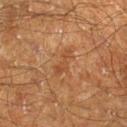This lesion was catalogued during total-body skin photography and was not selected for biopsy. The lesion is on the right lower leg. Automated image analysis of the tile measured a border-irregularity index near 6/10, internal color variation of about 1 on a 0–10 scale, and a peripheral color-asymmetry measure near 0. The software also gave lesion-presence confidence of about 100/100. A roughly 15 mm field-of-view crop from a total-body skin photograph. Measured at roughly 3.5 mm in maximum diameter. The patient is a male approximately 60 years of age.